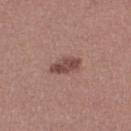biopsy status: imaged on a skin check; not biopsied | automated lesion analysis: a lesion area of about 6 mm², an eccentricity of roughly 0.85, and two-axis asymmetry of about 0.25; an average lesion color of about L≈46 a*≈21 b*≈23 (CIELAB), roughly 12 lightness units darker than nearby skin, and a lesion-to-skin contrast of about 8.5 (normalized; higher = more distinct); an automated nevus-likeness rating near 80 out of 100 and a detector confidence of about 100 out of 100 that the crop contains a lesion | lesion diameter: ≈3.5 mm | anatomic site: the left leg | patient: female, aged around 35 | imaging modality: 15 mm crop, total-body photography | tile lighting: white-light illumination.